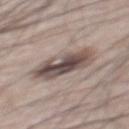Recorded during total-body skin imaging; not selected for excision or biopsy. A 15 mm close-up extracted from a 3D total-body photography capture. The total-body-photography lesion software estimated a nevus-likeness score of about 45/100 and a lesion-detection confidence of about 65/100. A male patient, aged 63 to 67. Measured at roughly 5.5 mm in maximum diameter. Imaged with white-light lighting. The lesion is located on the back.